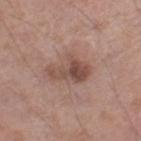Impression:
Part of a total-body skin-imaging series; this lesion was reviewed on a skin check and was not flagged for biopsy.
Background:
Longest diameter approximately 4.5 mm. The lesion is on the left lower leg. A 15 mm close-up extracted from a 3D total-body photography capture. The patient is a male roughly 55 years of age.